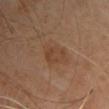The lesion was photographed on a routine skin check and not biopsied; there is no pathology result.
This is a cross-polarized tile.
An algorithmic analysis of the crop reported a footprint of about 7.5 mm² and an eccentricity of roughly 0.7. The analysis additionally found a lesion color around L≈42 a*≈19 b*≈30 in CIELAB, roughly 6 lightness units darker than nearby skin, and a normalized border contrast of about 5.5. The analysis additionally found a lesion-detection confidence of about 100/100.
On the chest.
Cropped from a whole-body photographic skin survey; the tile spans about 15 mm.
The subject is a female approximately 60 years of age.